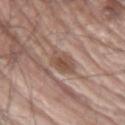- notes · no biopsy performed (imaged during a skin exam)
- diameter · about 3.5 mm
- image · total-body-photography crop, ~15 mm field of view
- location · the right upper arm
- lighting · white-light illumination
- subject · male, in their mid- to late 60s
- TBP lesion metrics · a footprint of about 6 mm², an eccentricity of roughly 0.7, and two-axis asymmetry of about 0.25; a border-irregularity index near 2.5/10, a color-variation rating of about 3.5/10, and peripheral color asymmetry of about 1; an automated nevus-likeness rating near 95 out of 100